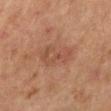No biopsy was performed on this lesion — it was imaged during a full skin examination and was not determined to be concerning.